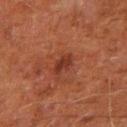{"biopsy_status": "not biopsied; imaged during a skin examination", "site": "left arm", "automated_metrics": {"area_mm2_approx": 3.0, "eccentricity": 0.75, "shape_asymmetry": 0.25, "cielab_L": 26, "cielab_a": 22, "cielab_b": 24, "border_irregularity_0_10": 2.5, "color_variation_0_10": 1.5}, "patient": {"sex": "male", "age_approx": 60}, "image": {"source": "total-body photography crop", "field_of_view_mm": 15}, "lesion_size": {"long_diameter_mm_approx": 2.5}, "lighting": "cross-polarized"}